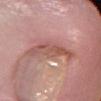From the left lower leg. The tile uses white-light illumination. Measured at roughly 6 mm in maximum diameter. A female subject, roughly 75 years of age. A 15 mm crop from a total-body photograph taken for skin-cancer surveillance.